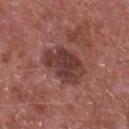notes — imaged on a skin check; not biopsied
subject — male, aged 63–67
automated lesion analysis — a border-irregularity index near 3/10 and radial color variation of about 1.5; a nevus-likeness score of about 10/100 and a detector confidence of about 100 out of 100 that the crop contains a lesion
body site — the chest
size — ≈5.5 mm
image source — total-body-photography crop, ~15 mm field of view
lighting — white-light illumination